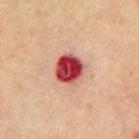image source — ~15 mm crop, total-body skin-cancer survey | anatomic site — the abdomen | lighting — cross-polarized illumination | patient — male, aged 63 to 67 | diameter — ~3.5 mm (longest diameter).